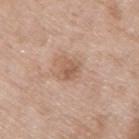Captured during whole-body skin photography for melanoma surveillance; the lesion was not biopsied.
A 15 mm close-up extracted from a 3D total-body photography capture.
A female patient about 75 years old.
The lesion is on the right upper arm.